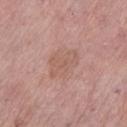Q: What is the anatomic site?
A: the left lower leg
Q: What is the lesion's diameter?
A: ≈4.5 mm
Q: Who is the patient?
A: female, aged 63 to 67
Q: What is the imaging modality?
A: ~15 mm tile from a whole-body skin photo